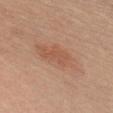workup — imaged on a skin check; not biopsied
tile lighting — white-light
anatomic site — the chest
imaging modality — 15 mm crop, total-body photography
patient — female, in their 20s
TBP lesion metrics — an area of roughly 13 mm², a shape eccentricity near 0.9, and two-axis asymmetry of about 0.2; an average lesion color of about L≈56 a*≈22 b*≈31 (CIELAB); an automated nevus-likeness rating near 85 out of 100 and a detector confidence of about 100 out of 100 that the crop contains a lesion
diameter — about 6.5 mm